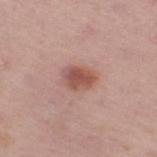No biopsy was performed on this lesion — it was imaged during a full skin examination and was not determined to be concerning. The total-body-photography lesion software estimated an average lesion color of about L≈53 a*≈24 b*≈25 (CIELAB), about 11 CIELAB-L* units darker than the surrounding skin, and a lesion-to-skin contrast of about 8 (normalized; higher = more distinct). The analysis additionally found a border-irregularity rating of about 1.5/10, a within-lesion color-variation index near 4.5/10, and a peripheral color-asymmetry measure near 1.5. The software also gave a classifier nevus-likeness of about 90/100 and lesion-presence confidence of about 100/100. A female patient, aged approximately 65. This image is a 15 mm lesion crop taken from a total-body photograph. Imaged with white-light lighting. Located on the right thigh. About 3.5 mm across.